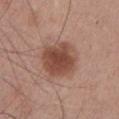Q: Was this lesion biopsied?
A: imaged on a skin check; not biopsied
Q: How was the tile lit?
A: white-light illumination
Q: Who is the patient?
A: male, aged approximately 25
Q: Automated lesion metrics?
A: an area of roughly 17 mm² and two-axis asymmetry of about 0.1; a normalized border contrast of about 9; border irregularity of about 1 on a 0–10 scale, a within-lesion color-variation index near 4.5/10, and radial color variation of about 1
Q: Lesion size?
A: ≈5 mm
Q: What is the anatomic site?
A: the chest
Q: How was this image acquired?
A: ~15 mm crop, total-body skin-cancer survey